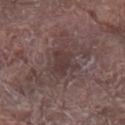  biopsy_status: not biopsied; imaged during a skin examination
  patient:
    sex: male
    age_approx: 80
  image:
    source: total-body photography crop
    field_of_view_mm: 15
  site: arm